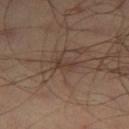Assessment:
No biopsy was performed on this lesion — it was imaged during a full skin examination and was not determined to be concerning.
Background:
Located on the left thigh. An algorithmic analysis of the crop reported a lesion area of about 2.5 mm², a shape eccentricity near 0.85, and a shape-asymmetry score of about 0.45 (0 = symmetric). And it measured a border-irregularity index near 5/10 and a color-variation rating of about 0/10. This image is a 15 mm lesion crop taken from a total-body photograph. A male patient, aged 43–47. This is a cross-polarized tile. Longest diameter approximately 2.5 mm.